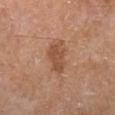  biopsy_status: not biopsied; imaged during a skin examination
  lesion_size:
    long_diameter_mm_approx: 4.0
  lighting: cross-polarized
  image:
    source: total-body photography crop
    field_of_view_mm: 15
  site: right lower leg
  automated_metrics:
    area_mm2_approx: 7.5
    shape_asymmetry: 0.35
  patient:
    sex: male
    age_approx: 65